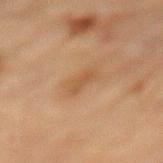The lesion was tiled from a total-body skin photograph and was not biopsied. A roughly 15 mm field-of-view crop from a total-body skin photograph. From the back. A female subject in their 80s. Imaged with cross-polarized lighting. Longest diameter approximately 3.5 mm.